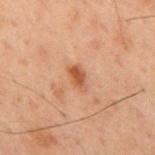<lesion>
<biopsy_status>not biopsied; imaged during a skin examination</biopsy_status>
<automated_metrics>
  <area_mm2_approx>3.5</area_mm2_approx>
  <eccentricity>0.8</eccentricity>
  <shape_asymmetry>0.25</shape_asymmetry>
  <cielab_L>41</cielab_L>
  <cielab_a>21</cielab_a>
  <cielab_b>29</cielab_b>
  <vs_skin_contrast_norm>8.5</vs_skin_contrast_norm>
</automated_metrics>
<lighting>cross-polarized</lighting>
<site>mid back</site>
<patient>
  <sex>male</sex>
  <age_approx>60</age_approx>
</patient>
<image>
  <source>total-body photography crop</source>
  <field_of_view_mm>15</field_of_view_mm>
</image>
</lesion>